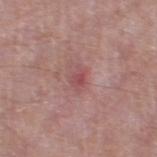Recorded during total-body skin imaging; not selected for excision or biopsy. The recorded lesion diameter is about 2.5 mm. The total-body-photography lesion software estimated an eccentricity of roughly 0.8 and two-axis asymmetry of about 0.25. It also reported a border-irregularity rating of about 3/10, internal color variation of about 3.5 on a 0–10 scale, and a peripheral color-asymmetry measure near 1.5. A male patient, approximately 65 years of age. The lesion is on the left thigh. The tile uses white-light illumination. A close-up tile cropped from a whole-body skin photograph, about 15 mm across.